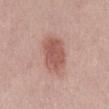<case>
  <patient>
    <sex>male</sex>
    <age_approx>70</age_approx>
  </patient>
  <lesion_size>
    <long_diameter_mm_approx>5.0</long_diameter_mm_approx>
  </lesion_size>
  <site>abdomen</site>
  <image>
    <source>total-body photography crop</source>
    <field_of_view_mm>15</field_of_view_mm>
  </image>
  <lighting>white-light</lighting>
</case>